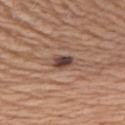Clinical impression:
Captured during whole-body skin photography for melanoma surveillance; the lesion was not biopsied.
Background:
The tile uses white-light illumination. From the upper back. Cropped from a whole-body photographic skin survey; the tile spans about 15 mm. A male patient, roughly 65 years of age.